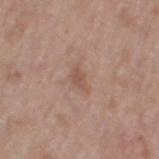notes — no biopsy performed (imaged during a skin exam) | TBP lesion metrics — an area of roughly 3.5 mm² and a symmetry-axis asymmetry near 0.4; a border-irregularity index near 4/10 and a peripheral color-asymmetry measure near 0.5; a nevus-likeness score of about 0/100 | subject — female, aged around 60 | acquisition — ~15 mm tile from a whole-body skin photo | lesion size — ~3 mm (longest diameter) | site — the left thigh.